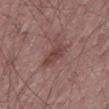{
  "biopsy_status": "not biopsied; imaged during a skin examination",
  "image": {
    "source": "total-body photography crop",
    "field_of_view_mm": 15
  },
  "patient": {
    "sex": "male",
    "age_approx": 65
  },
  "lighting": "white-light",
  "site": "lower back",
  "lesion_size": {
    "long_diameter_mm_approx": 3.5
  }
}